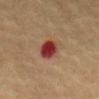Context: Imaged with cross-polarized lighting. Cropped from a whole-body photographic skin survey; the tile spans about 15 mm. On the front of the torso. The lesion's longest dimension is about 3 mm. A female subject in their mid-50s.